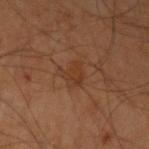Acquisition and patient details: The total-body-photography lesion software estimated a footprint of about 4.5 mm² and a symmetry-axis asymmetry near 0.3. And it measured a lesion color around L≈32 a*≈19 b*≈29 in CIELAB, a lesion–skin lightness drop of about 5, and a normalized lesion–skin contrast near 5.5. The software also gave a border-irregularity index near 3/10 and peripheral color asymmetry of about 1. About 2.5 mm across. The lesion is on the right upper arm. Imaged with cross-polarized lighting. A 15 mm close-up tile from a total-body photography series done for melanoma screening. A male subject, roughly 60 years of age.